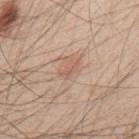A male subject aged 48–52.
From the upper back.
A close-up tile cropped from a whole-body skin photograph, about 15 mm across.